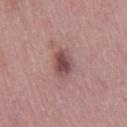{"lighting": "white-light", "image": {"source": "total-body photography crop", "field_of_view_mm": 15}, "lesion_size": {"long_diameter_mm_approx": 4.0}, "patient": {"sex": "female", "age_approx": 50}, "site": "right thigh"}